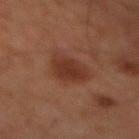<case>
  <biopsy_status>not biopsied; imaged during a skin examination</biopsy_status>
  <lighting>cross-polarized</lighting>
  <site>front of the torso</site>
  <image>
    <source>total-body photography crop</source>
    <field_of_view_mm>15</field_of_view_mm>
  </image>
  <automated_metrics>
    <area_mm2_approx>11.0</area_mm2_approx>
    <shape_asymmetry>0.25</shape_asymmetry>
    <cielab_L>31</cielab_L>
    <cielab_a>21</cielab_a>
    <cielab_b>26</cielab_b>
    <vs_skin_darker_L>8.0</vs_skin_darker_L>
    <vs_skin_contrast_norm>7.5</vs_skin_contrast_norm>
    <nevus_likeness_0_100>95</nevus_likeness_0_100>
    <lesion_detection_confidence_0_100>100</lesion_detection_confidence_0_100>
  </automated_metrics>
  <lesion_size>
    <long_diameter_mm_approx>5.0</long_diameter_mm_approx>
  </lesion_size>
  <patient>
    <sex>male</sex>
    <age_approx>60</age_approx>
  </patient>
</case>